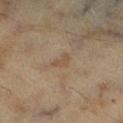Notes:
– biopsy status: catalogued during a skin exam; not biopsied